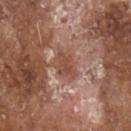Q: Is there a histopathology result?
A: total-body-photography surveillance lesion; no biopsy
Q: How was the tile lit?
A: white-light illumination
Q: What kind of image is this?
A: 15 mm crop, total-body photography
Q: What did automated image analysis measure?
A: an area of roughly 5.5 mm², an outline eccentricity of about 0.8 (0 = round, 1 = elongated), and a shape-asymmetry score of about 0.25 (0 = symmetric); a normalized border contrast of about 6; a border-irregularity index near 3/10
Q: Who is the patient?
A: male, aged 78 to 82
Q: What is the anatomic site?
A: the head or neck
Q: Lesion size?
A: about 3.5 mm